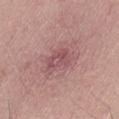Recorded during total-body skin imaging; not selected for excision or biopsy. From the left thigh. Measured at roughly 2.5 mm in maximum diameter. Cropped from a total-body skin-imaging series; the visible field is about 15 mm. The patient is a male aged 43 to 47. Captured under white-light illumination.